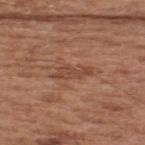The lesion was photographed on a routine skin check and not biopsied; there is no pathology result. Measured at roughly 4 mm in maximum diameter. A male patient, about 55 years old. A 15 mm close-up tile from a total-body photography series done for melanoma screening. The lesion is located on the upper back.